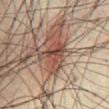Imaged during a routine full-body skin examination; the lesion was not biopsied and no histopathology is available. Automated tile analysis of the lesion measured a footprint of about 8.5 mm², an outline eccentricity of about 0.55 (0 = round, 1 = elongated), and a symmetry-axis asymmetry near 0.6. And it measured border irregularity of about 6.5 on a 0–10 scale, internal color variation of about 7.5 on a 0–10 scale, and a peripheral color-asymmetry measure near 3. And it measured lesion-presence confidence of about 85/100. Located on the abdomen. A close-up tile cropped from a whole-body skin photograph, about 15 mm across. A male patient in their 50s. This is a cross-polarized tile. Longest diameter approximately 4.5 mm.